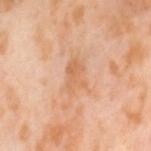Assessment:
Captured during whole-body skin photography for melanoma surveillance; the lesion was not biopsied.
Background:
The lesion's longest dimension is about 4 mm. This image is a 15 mm lesion crop taken from a total-body photograph. The lesion is on the left thigh. The patient is a female aged 53 to 57. This is a cross-polarized tile.